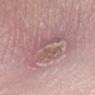workup: imaged on a skin check; not biopsied | tile lighting: white-light | subject: female, in their 40s | TBP lesion metrics: an eccentricity of roughly 0.8 and a shape-asymmetry score of about 0.35 (0 = symmetric); roughly 8 lightness units darker than nearby skin and a lesion-to-skin contrast of about 5 (normalized; higher = more distinct); a border-irregularity index near 7.5/10, a within-lesion color-variation index near 6.5/10, and peripheral color asymmetry of about 2; an automated nevus-likeness rating near 0 out of 100 and a detector confidence of about 95 out of 100 that the crop contains a lesion | acquisition: 15 mm crop, total-body photography | location: the left lower leg | lesion size: about 8.5 mm.